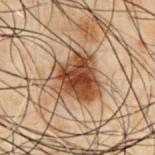subject — male, aged 48–52; image — ~15 mm tile from a whole-body skin photo; site — the chest.